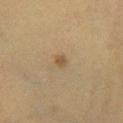Clinical impression:
Part of a total-body skin-imaging series; this lesion was reviewed on a skin check and was not flagged for biopsy.
Context:
A female subject, aged 38 to 42. A lesion tile, about 15 mm wide, cut from a 3D total-body photograph. The lesion is located on the right lower leg. Automated image analysis of the tile measured a shape eccentricity near 0.55 and two-axis asymmetry of about 0.25. It also reported a lesion color around L≈43 a*≈13 b*≈31 in CIELAB, roughly 8 lightness units darker than nearby skin, and a normalized lesion–skin contrast near 7. And it measured a border-irregularity index near 2/10, internal color variation of about 0.5 on a 0–10 scale, and peripheral color asymmetry of about 0. And it measured an automated nevus-likeness rating near 75 out of 100. This is a cross-polarized tile.